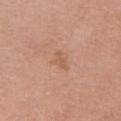The lesion was photographed on a routine skin check and not biopsied; there is no pathology result. A female subject, aged around 60. On the chest. Captured under white-light illumination. A 15 mm close-up tile from a total-body photography series done for melanoma screening.